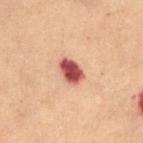<case>
  <automated_metrics>
    <area_mm2_approx>6.0</area_mm2_approx>
    <shape_asymmetry>0.15</shape_asymmetry>
    <nevus_likeness_0_100>15</nevus_likeness_0_100>
    <lesion_detection_confidence_0_100>100</lesion_detection_confidence_0_100>
  </automated_metrics>
  <lighting>cross-polarized</lighting>
  <site>lower back</site>
  <image>
    <source>total-body photography crop</source>
    <field_of_view_mm>15</field_of_view_mm>
  </image>
  <patient>
    <sex>female</sex>
    <age_approx>80</age_approx>
  </patient>
  <lesion_size>
    <long_diameter_mm_approx>3.5</long_diameter_mm_approx>
  </lesion_size>
</case>